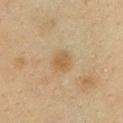{"biopsy_status": "not biopsied; imaged during a skin examination", "lighting": "cross-polarized", "patient": {"sex": "female", "age_approx": 40}, "lesion_size": {"long_diameter_mm_approx": 2.5}, "automated_metrics": {"vs_skin_darker_L": 6.0, "nevus_likeness_0_100": 50, "lesion_detection_confidence_0_100": 100}, "site": "chest", "image": {"source": "total-body photography crop", "field_of_view_mm": 15}}